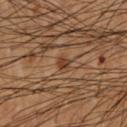Notes:
– workup — total-body-photography surveillance lesion; no biopsy
– image source — ~15 mm crop, total-body skin-cancer survey
– site — the chest
– subject — male, about 55 years old
– size — ~1.5 mm (longest diameter)